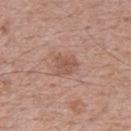{
  "lighting": "white-light",
  "patient": {
    "sex": "male",
    "age_approx": 70
  },
  "site": "back",
  "automated_metrics": {
    "area_mm2_approx": 5.5,
    "eccentricity": 0.55,
    "cielab_L": 54,
    "cielab_a": 22,
    "cielab_b": 27,
    "vs_skin_darker_L": 8.0,
    "border_irregularity_0_10": 3.5,
    "color_variation_0_10": 2.5,
    "peripheral_color_asymmetry": 1.0,
    "nevus_likeness_0_100": 0
  },
  "image": {
    "source": "total-body photography crop",
    "field_of_view_mm": 15
  }
}